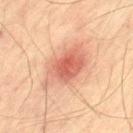Assessment: Imaged during a routine full-body skin examination; the lesion was not biopsied and no histopathology is available. Clinical summary: A male patient, aged 73–77. Cropped from a whole-body photographic skin survey; the tile spans about 15 mm. About 6 mm across. Located on the left thigh.